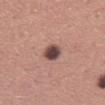| key | value |
|---|---|
| notes | total-body-photography surveillance lesion; no biopsy |
| imaging modality | ~15 mm tile from a whole-body skin photo |
| patient | male, aged around 45 |
| location | the mid back |
| automated metrics | a lesion area of about 5 mm²; a border-irregularity rating of about 1.5/10, a color-variation rating of about 6/10, and radial color variation of about 2 |
| diameter | ≈3 mm |
| lighting | white-light illumination |